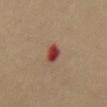Clinical impression: This lesion was catalogued during total-body skin photography and was not selected for biopsy. Context: The patient is a female about 35 years old. The tile uses cross-polarized illumination. The recorded lesion diameter is about 3 mm. A roughly 15 mm field-of-view crop from a total-body skin photograph. The lesion is located on the abdomen.